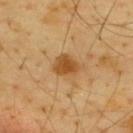<record>
  <biopsy_status>not biopsied; imaged during a skin examination</biopsy_status>
  <lighting>cross-polarized</lighting>
  <site>upper back</site>
  <image>
    <source>total-body photography crop</source>
    <field_of_view_mm>15</field_of_view_mm>
  </image>
  <lesion_size>
    <long_diameter_mm_approx>3.0</long_diameter_mm_approx>
  </lesion_size>
  <patient>
    <sex>male</sex>
    <age_approx>65</age_approx>
  </patient>
</record>